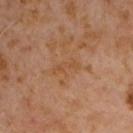Recorded during total-body skin imaging; not selected for excision or biopsy. From the chest. Captured under cross-polarized illumination. A close-up tile cropped from a whole-body skin photograph, about 15 mm across. About 3.5 mm across. A male subject, aged approximately 60.